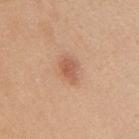follow-up = no biopsy performed (imaged during a skin exam)
subject = female, aged approximately 40
imaging modality = total-body-photography crop, ~15 mm field of view
size = ~3 mm (longest diameter)
location = the mid back